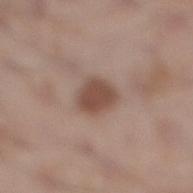biopsy_status: not biopsied; imaged during a skin examination
lesion_size:
  long_diameter_mm_approx: 4.0
automated_metrics:
  area_mm2_approx: 8.0
  eccentricity: 0.7
  shape_asymmetry: 0.2
  cielab_L: 48
  cielab_a: 18
  cielab_b: 25
  vs_skin_darker_L: 12.0
  vs_skin_contrast_norm: 9.0
  border_irregularity_0_10: 2.0
  peripheral_color_asymmetry: 0.5
patient:
  sex: male
  age_approx: 35
site: right lower leg
lighting: white-light
image:
  source: total-body photography crop
  field_of_view_mm: 15